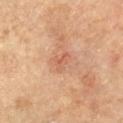Assessment: The lesion was photographed on a routine skin check and not biopsied; there is no pathology result. Clinical summary: Measured at roughly 2.5 mm in maximum diameter. A female subject aged around 65. On the arm. A 15 mm crop from a total-body photograph taken for skin-cancer surveillance. The tile uses cross-polarized illumination.